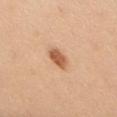imaging modality = 15 mm crop, total-body photography | subject = male, roughly 35 years of age | location = the upper back.